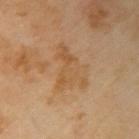Captured during whole-body skin photography for melanoma surveillance; the lesion was not biopsied. The total-body-photography lesion software estimated a lesion area of about 15 mm², an outline eccentricity of about 0.75 (0 = round, 1 = elongated), and two-axis asymmetry of about 0.5. And it measured an average lesion color of about L≈55 a*≈18 b*≈38 (CIELAB), about 5 CIELAB-L* units darker than the surrounding skin, and a normalized lesion–skin contrast near 5. And it measured a detector confidence of about 100 out of 100 that the crop contains a lesion. The lesion's longest dimension is about 5.5 mm. A male subject, aged approximately 65. A roughly 15 mm field-of-view crop from a total-body skin photograph. The lesion is on the right upper arm. Captured under cross-polarized illumination.